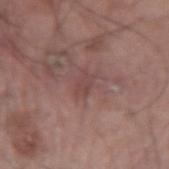Assessment:
The lesion was tiled from a total-body skin photograph and was not biopsied.
Clinical summary:
A male patient in their mid- to late 60s. Cropped from a total-body skin-imaging series; the visible field is about 15 mm. This is a white-light tile. The total-body-photography lesion software estimated an outline eccentricity of about 0.85 (0 = round, 1 = elongated). And it measured an automated nevus-likeness rating near 0 out of 100 and a lesion-detection confidence of about 70/100. Measured at roughly 3 mm in maximum diameter. From the right upper arm.